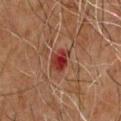Impression: The lesion was tiled from a total-body skin photograph and was not biopsied. Background: Longest diameter approximately 2.5 mm. The lesion is on the chest. The tile uses cross-polarized illumination. A male patient in their mid- to late 60s. A region of skin cropped from a whole-body photographic capture, roughly 15 mm wide.